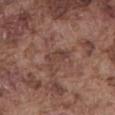Clinical impression:
Imaged during a routine full-body skin examination; the lesion was not biopsied and no histopathology is available.
Acquisition and patient details:
A male subject, roughly 75 years of age. Longest diameter approximately 3.5 mm. From the front of the torso. Cropped from a whole-body photographic skin survey; the tile spans about 15 mm.